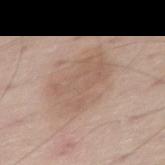biopsy_status: not biopsied; imaged during a skin examination
image:
  source: total-body photography crop
  field_of_view_mm: 15
site: right thigh
lesion_size:
  long_diameter_mm_approx: 6.0
lighting: white-light
patient:
  sex: male
  age_approx: 60